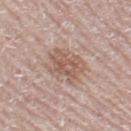This lesion was catalogued during total-body skin photography and was not selected for biopsy.
The lesion's longest dimension is about 5 mm.
Located on the left thigh.
A lesion tile, about 15 mm wide, cut from a 3D total-body photograph.
The subject is a female in their mid- to late 40s.
Captured under white-light illumination.